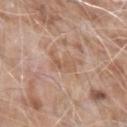Q: Was this lesion biopsied?
A: catalogued during a skin exam; not biopsied
Q: How was this image acquired?
A: ~15 mm crop, total-body skin-cancer survey
Q: What is the anatomic site?
A: the left forearm
Q: Patient demographics?
A: male, in their mid-70s